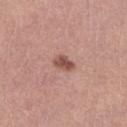| field | value |
|---|---|
| notes | imaged on a skin check; not biopsied |
| lesion size | ≈2.5 mm |
| subject | female, aged approximately 60 |
| imaging modality | total-body-photography crop, ~15 mm field of view |
| location | the left lower leg |
| TBP lesion metrics | a footprint of about 4 mm², a shape eccentricity near 0.7, and a shape-asymmetry score of about 0.25 (0 = symmetric); a lesion color around L≈51 a*≈24 b*≈25 in CIELAB, a lesion–skin lightness drop of about 13, and a lesion-to-skin contrast of about 8.5 (normalized; higher = more distinct); lesion-presence confidence of about 100/100 |